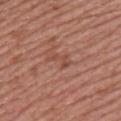biopsy status = total-body-photography surveillance lesion; no biopsy.